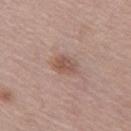follow-up: imaged on a skin check; not biopsied
subject: female, aged approximately 60
lesion diameter: ≈3 mm
body site: the left thigh
TBP lesion metrics: border irregularity of about 1.5 on a 0–10 scale, internal color variation of about 3 on a 0–10 scale, and a peripheral color-asymmetry measure near 1; an automated nevus-likeness rating near 25 out of 100 and a lesion-detection confidence of about 100/100
imaging modality: total-body-photography crop, ~15 mm field of view
lighting: white-light illumination